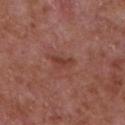{"image": {"source": "total-body photography crop", "field_of_view_mm": 15}, "lighting": "white-light", "lesion_size": {"long_diameter_mm_approx": 3.0}, "site": "chest", "automated_metrics": {"nevus_likeness_0_100": 0, "lesion_detection_confidence_0_100": 100}, "patient": {"sex": "male", "age_approx": 65}}